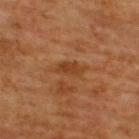<lesion>
  <biopsy_status>not biopsied; imaged during a skin examination</biopsy_status>
  <lesion_size>
    <long_diameter_mm_approx>3.5</long_diameter_mm_approx>
  </lesion_size>
  <site>back</site>
  <patient>
    <sex>male</sex>
    <age_approx>65</age_approx>
  </patient>
  <lighting>cross-polarized</lighting>
  <image>
    <source>total-body photography crop</source>
    <field_of_view_mm>15</field_of_view_mm>
  </image>
</lesion>